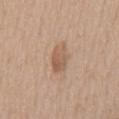The lesion was tiled from a total-body skin photograph and was not biopsied.
On the chest.
The patient is a male in their mid-60s.
Cropped from a whole-body photographic skin survey; the tile spans about 15 mm.